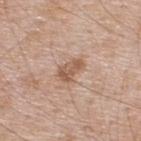  biopsy_status: not biopsied; imaged during a skin examination
  lesion_size:
    long_diameter_mm_approx: 3.0
  site: upper back
  image:
    source: total-body photography crop
    field_of_view_mm: 15
  lighting: white-light
  patient:
    sex: male
    age_approx: 50